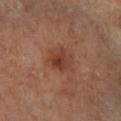{"biopsy_status": "not biopsied; imaged during a skin examination", "image": {"source": "total-body photography crop", "field_of_view_mm": 15}, "patient": {"sex": "male", "age_approx": 70}, "lighting": "cross-polarized", "site": "left leg", "automated_metrics": {"area_mm2_approx": 6.0, "eccentricity": 0.5, "shape_asymmetry": 0.25, "lesion_detection_confidence_0_100": 100}}